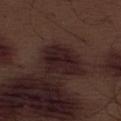• biopsy status: total-body-photography surveillance lesion; no biopsy
• lesion size: ≈5 mm
• tile lighting: white-light illumination
• acquisition: ~15 mm crop, total-body skin-cancer survey
• subject: male, in their 70s
• anatomic site: the abdomen